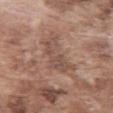  biopsy_status: not biopsied; imaged during a skin examination
  lighting: white-light
  image:
    source: total-body photography crop
    field_of_view_mm: 15
  patient:
    sex: male
    age_approx: 75
  site: abdomen